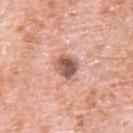The lesion was photographed on a routine skin check and not biopsied; there is no pathology result. On the upper back. Longest diameter approximately 3 mm. The lesion-visualizer software estimated a mean CIELAB color near L≈57 a*≈24 b*≈26 and a lesion-to-skin contrast of about 9.5 (normalized; higher = more distinct). The analysis additionally found border irregularity of about 2 on a 0–10 scale, a color-variation rating of about 6.5/10, and radial color variation of about 2. And it measured a nevus-likeness score of about 45/100 and a detector confidence of about 100 out of 100 that the crop contains a lesion. A lesion tile, about 15 mm wide, cut from a 3D total-body photograph. A male patient aged 78 to 82. Imaged with white-light lighting.